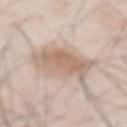Q: Was this lesion biopsied?
A: catalogued during a skin exam; not biopsied
Q: Patient demographics?
A: male, in their mid-50s
Q: What did automated image analysis measure?
A: a lesion color around L≈65 a*≈15 b*≈26 in CIELAB and a normalized lesion–skin contrast near 6.5
Q: Where on the body is the lesion?
A: the abdomen
Q: Illumination type?
A: white-light
Q: How was this image acquired?
A: ~15 mm crop, total-body skin-cancer survey